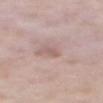Image and clinical context: The subject is a male aged 58–62. Measured at roughly 2.5 mm in maximum diameter. Imaged with white-light lighting. The total-body-photography lesion software estimated roughly 8 lightness units darker than nearby skin and a normalized border contrast of about 5.5. It also reported border irregularity of about 2.5 on a 0–10 scale, a within-lesion color-variation index near 0/10, and peripheral color asymmetry of about 0. And it measured a nevus-likeness score of about 0/100 and lesion-presence confidence of about 100/100. From the mid back. A roughly 15 mm field-of-view crop from a total-body skin photograph.